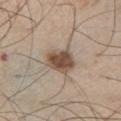Clinical impression: The lesion was photographed on a routine skin check and not biopsied; there is no pathology result. Image and clinical context: This image is a 15 mm lesion crop taken from a total-body photograph. The lesion's longest dimension is about 4 mm. Captured under white-light illumination. A male patient aged approximately 60. Located on the left thigh. Automated tile analysis of the lesion measured a lesion-to-skin contrast of about 10.5 (normalized; higher = more distinct). And it measured a within-lesion color-variation index near 3.5/10 and peripheral color asymmetry of about 1.